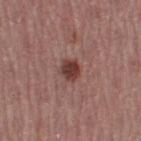<case>
<biopsy_status>not biopsied; imaged during a skin examination</biopsy_status>
<patient>
  <sex>female</sex>
  <age_approx>55</age_approx>
</patient>
<image>
  <source>total-body photography crop</source>
  <field_of_view_mm>15</field_of_view_mm>
</image>
<lesion_size>
  <long_diameter_mm_approx>2.5</long_diameter_mm_approx>
</lesion_size>
<lighting>white-light</lighting>
<site>leg</site>
</case>